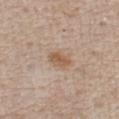Part of a total-body skin-imaging series; this lesion was reviewed on a skin check and was not flagged for biopsy. A region of skin cropped from a whole-body photographic capture, roughly 15 mm wide. From the chest. The patient is a male aged 73 to 77.